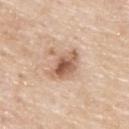Imaged during a routine full-body skin examination; the lesion was not biopsied and no histopathology is available. A roughly 15 mm field-of-view crop from a total-body skin photograph. An algorithmic analysis of the crop reported an average lesion color of about L≈60 a*≈19 b*≈31 (CIELAB) and a normalized lesion–skin contrast near 8.5. And it measured a within-lesion color-variation index near 7/10 and radial color variation of about 2.5. And it measured a classifier nevus-likeness of about 75/100 and lesion-presence confidence of about 100/100. On the upper back. The subject is a male roughly 80 years of age.